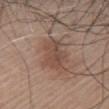notes=catalogued during a skin exam; not biopsied | acquisition=~15 mm tile from a whole-body skin photo | location=the chest | subject=male, aged approximately 60 | lesion diameter=about 5.5 mm | illumination=white-light illumination.